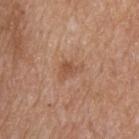  biopsy_status: not biopsied; imaged during a skin examination
  automated_metrics:
    area_mm2_approx: 4.5
    eccentricity: 0.75
    cielab_L: 52
    cielab_a: 22
    cielab_b: 32
    vs_skin_darker_L: 8.0
    border_irregularity_0_10: 4.5
    color_variation_0_10: 2.5
    peripheral_color_asymmetry: 0.5
  lighting: white-light
  site: upper back
  image:
    source: total-body photography crop
    field_of_view_mm: 15
  lesion_size:
    long_diameter_mm_approx: 3.0
  patient:
    sex: male
    age_approx: 55